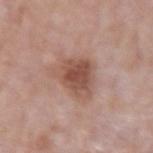<record>
  <biopsy_status>not biopsied; imaged during a skin examination</biopsy_status>
  <site>right forearm</site>
  <image>
    <source>total-body photography crop</source>
    <field_of_view_mm>15</field_of_view_mm>
  </image>
  <patient>
    <sex>female</sex>
    <age_approx>70</age_approx>
  </patient>
</record>